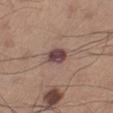| feature | finding |
|---|---|
| biopsy status | catalogued during a skin exam; not biopsied |